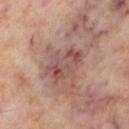Imaged during a routine full-body skin examination; the lesion was not biopsied and no histopathology is available.
Imaged with cross-polarized lighting.
A female patient aged 53–57.
From the left lower leg.
This image is a 15 mm lesion crop taken from a total-body photograph.
Automated tile analysis of the lesion measured a mean CIELAB color near L≈51 a*≈20 b*≈23, a lesion–skin lightness drop of about 9, and a normalized lesion–skin contrast near 7. The software also gave border irregularity of about 3.5 on a 0–10 scale, a color-variation rating of about 6.5/10, and a peripheral color-asymmetry measure near 2. And it measured a nevus-likeness score of about 0/100 and lesion-presence confidence of about 100/100.
The recorded lesion diameter is about 5.5 mm.